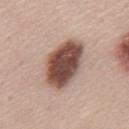Assessment:
Recorded during total-body skin imaging; not selected for excision or biopsy.
Image and clinical context:
The lesion-visualizer software estimated a border-irregularity rating of about 1.5/10, a within-lesion color-variation index near 5.5/10, and a peripheral color-asymmetry measure near 1.5. It also reported a classifier nevus-likeness of about 70/100 and lesion-presence confidence of about 100/100. Located on the mid back. A 15 mm close-up extracted from a 3D total-body photography capture. A male subject, aged 43 to 47. Longest diameter approximately 6.5 mm.